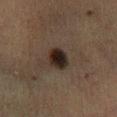On the left lower leg.
This is a cross-polarized tile.
Automated image analysis of the tile measured a footprint of about 6.5 mm² and a symmetry-axis asymmetry near 0.15.
A female patient, roughly 55 years of age.
Cropped from a whole-body photographic skin survey; the tile spans about 15 mm.
Measured at roughly 3.5 mm in maximum diameter.